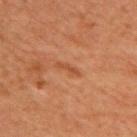The lesion was photographed on a routine skin check and not biopsied; there is no pathology result.
A female patient aged approximately 40.
The lesion is located on the upper back.
Cropped from a whole-body photographic skin survey; the tile spans about 15 mm.
The tile uses cross-polarized illumination.
The lesion-visualizer software estimated an eccentricity of roughly 0.95 and a symmetry-axis asymmetry near 0.5. And it measured a mean CIELAB color near L≈43 a*≈23 b*≈33 and a lesion-to-skin contrast of about 6 (normalized; higher = more distinct).